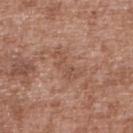No biopsy was performed on this lesion — it was imaged during a full skin examination and was not determined to be concerning. The tile uses white-light illumination. The lesion is located on the upper back. An algorithmic analysis of the crop reported a lesion color around L≈51 a*≈21 b*≈28 in CIELAB, a lesion–skin lightness drop of about 6, and a normalized lesion–skin contrast near 5. And it measured a classifier nevus-likeness of about 0/100 and a lesion-detection confidence of about 100/100. Longest diameter approximately 4 mm. A male subject aged 43–47. This image is a 15 mm lesion crop taken from a total-body photograph.